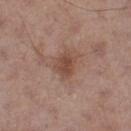Impression:
The lesion was tiled from a total-body skin photograph and was not biopsied.
Context:
The tile uses white-light illumination. The lesion is located on the leg. Measured at roughly 3 mm in maximum diameter. A 15 mm close-up tile from a total-body photography series done for melanoma screening. A male subject, aged 68–72. An algorithmic analysis of the crop reported a lesion area of about 6 mm² and an eccentricity of roughly 0.65. It also reported border irregularity of about 2 on a 0–10 scale, a within-lesion color-variation index near 2/10, and radial color variation of about 1. The software also gave a nevus-likeness score of about 50/100 and lesion-presence confidence of about 100/100.